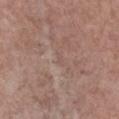Findings:
• follow-up — imaged on a skin check; not biopsied
• tile lighting — white-light illumination
• diameter — about 1 mm
• location — the chest
• image source — ~15 mm crop, total-body skin-cancer survey
• patient — female, aged approximately 65